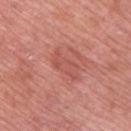{
  "biopsy_status": "not biopsied; imaged during a skin examination",
  "patient": {
    "sex": "male",
    "age_approx": 60
  },
  "lesion_size": {
    "long_diameter_mm_approx": 4.5
  },
  "site": "upper back",
  "image": {
    "source": "total-body photography crop",
    "field_of_view_mm": 15
  }
}